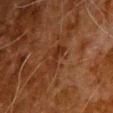{
  "biopsy_status": "not biopsied; imaged during a skin examination",
  "lighting": "cross-polarized",
  "patient": {
    "sex": "male",
    "age_approx": 80
  },
  "automated_metrics": {
    "cielab_L": 24,
    "cielab_a": 20,
    "cielab_b": 26,
    "vs_skin_darker_L": 5.0,
    "vs_skin_contrast_norm": 6.5,
    "border_irregularity_0_10": 5.5,
    "color_variation_0_10": 1.0,
    "peripheral_color_asymmetry": 0.5
  },
  "image": {
    "source": "total-body photography crop",
    "field_of_view_mm": 15
  },
  "site": "front of the torso"
}